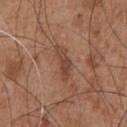Clinical impression: No biopsy was performed on this lesion — it was imaged during a full skin examination and was not determined to be concerning. Context: Imaged with white-light lighting. The subject is a male roughly 55 years of age. The total-body-photography lesion software estimated a color-variation rating of about 3.5/10 and peripheral color asymmetry of about 1. Longest diameter approximately 4.5 mm. A 15 mm close-up tile from a total-body photography series done for melanoma screening. The lesion is on the chest.